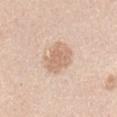notes = no biopsy performed (imaged during a skin exam) | size = ~4.5 mm (longest diameter) | patient = male, approximately 45 years of age | anatomic site = the right upper arm | image source = ~15 mm tile from a whole-body skin photo.